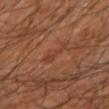The lesion was tiled from a total-body skin photograph and was not biopsied.